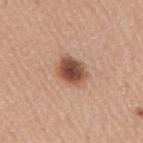Recorded during total-body skin imaging; not selected for excision or biopsy. Imaged with white-light lighting. The lesion is on the right upper arm. A roughly 15 mm field-of-view crop from a total-body skin photograph. The subject is a male aged 38–42. The lesion's longest dimension is about 3.5 mm.